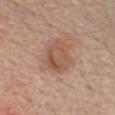<tbp_lesion>
<biopsy_status>not biopsied; imaged during a skin examination</biopsy_status>
<lighting>white-light</lighting>
<image>
  <source>total-body photography crop</source>
  <field_of_view_mm>15</field_of_view_mm>
</image>
<patient>
  <sex>male</sex>
  <age_approx>70</age_approx>
</patient>
<site>chest</site>
<automated_metrics>
  <border_irregularity_0_10>2.5</border_irregularity_0_10>
  <color_variation_0_10>4.5</color_variation_0_10>
  <peripheral_color_asymmetry>1.5</peripheral_color_asymmetry>
</automated_metrics>
<lesion_size>
  <long_diameter_mm_approx>4.0</long_diameter_mm_approx>
</lesion_size>
</tbp_lesion>